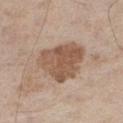Clinical impression:
The lesion was tiled from a total-body skin photograph and was not biopsied.
Image and clinical context:
Captured under white-light illumination. This image is a 15 mm lesion crop taken from a total-body photograph. Longest diameter approximately 5.5 mm. Located on the right thigh. The patient is a male aged 58 to 62. An algorithmic analysis of the crop reported border irregularity of about 3 on a 0–10 scale, a color-variation rating of about 3.5/10, and peripheral color asymmetry of about 1.5.